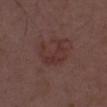{
  "biopsy_status": "not biopsied; imaged during a skin examination",
  "image": {
    "source": "total-body photography crop",
    "field_of_view_mm": 15
  },
  "lighting": "white-light",
  "patient": {
    "sex": "male",
    "age_approx": 55
  },
  "site": "front of the torso"
}